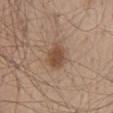| field | value |
|---|---|
| body site | the lower back |
| diameter | ~3 mm (longest diameter) |
| illumination | white-light illumination |
| subject | male, about 45 years old |
| image source | total-body-photography crop, ~15 mm field of view |